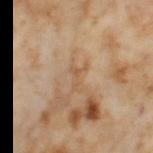notes=total-body-photography surveillance lesion; no biopsy | image source=total-body-photography crop, ~15 mm field of view | subject=female, roughly 55 years of age | size=≈2.5 mm | anatomic site=the left thigh.